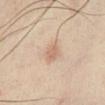workup=total-body-photography surveillance lesion; no biopsy | imaging modality=15 mm crop, total-body photography | patient=male, roughly 40 years of age | anatomic site=the right lower leg | lighting=cross-polarized.